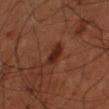biopsy status: catalogued during a skin exam; not biopsied
lighting: cross-polarized
automated lesion analysis: a lesion color around L≈20 a*≈18 b*≈21 in CIELAB and a lesion-to-skin contrast of about 8.5 (normalized; higher = more distinct); internal color variation of about 2 on a 0–10 scale; a nevus-likeness score of about 85/100 and lesion-presence confidence of about 100/100
subject: male, aged 68 to 72
lesion size: about 3 mm
location: the right forearm
image: ~15 mm crop, total-body skin-cancer survey